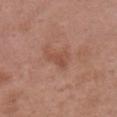Impression:
Recorded during total-body skin imaging; not selected for excision or biopsy.
Image and clinical context:
About 3.5 mm across. From the chest. Imaged with white-light lighting. An algorithmic analysis of the crop reported a footprint of about 5 mm², an eccentricity of roughly 0.8, and a shape-asymmetry score of about 0.5 (0 = symmetric). And it measured a lesion–skin lightness drop of about 7 and a normalized lesion–skin contrast near 5.5. It also reported a border-irregularity rating of about 5/10 and peripheral color asymmetry of about 1. It also reported an automated nevus-likeness rating near 0 out of 100 and a lesion-detection confidence of about 100/100. A female subject, in their mid- to late 60s. A 15 mm close-up tile from a total-body photography series done for melanoma screening.